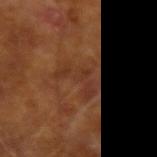notes: no biopsy performed (imaged during a skin exam)
image source: total-body-photography crop, ~15 mm field of view
location: the left upper arm
illumination: cross-polarized
diameter: ≈4.5 mm
automated lesion analysis: an average lesion color of about L≈33 a*≈21 b*≈29 (CIELAB) and a lesion-to-skin contrast of about 5 (normalized; higher = more distinct); a classifier nevus-likeness of about 0/100 and a detector confidence of about 95 out of 100 that the crop contains a lesion
patient: male, about 65 years old